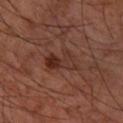Assessment: The lesion was tiled from a total-body skin photograph and was not biopsied. Image and clinical context: Captured under cross-polarized illumination. On the right forearm. A male patient in their mid-60s. Cropped from a total-body skin-imaging series; the visible field is about 15 mm.